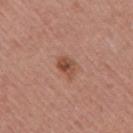Q: Is there a histopathology result?
A: total-body-photography surveillance lesion; no biopsy
Q: Lesion location?
A: the arm
Q: Patient demographics?
A: female, aged 53 to 57
Q: What lighting was used for the tile?
A: white-light illumination
Q: Automated lesion metrics?
A: an average lesion color of about L≈49 a*≈23 b*≈29 (CIELAB), a lesion–skin lightness drop of about 10, and a lesion-to-skin contrast of about 7.5 (normalized; higher = more distinct)
Q: What is the lesion's diameter?
A: about 3 mm
Q: What is the imaging modality?
A: total-body-photography crop, ~15 mm field of view